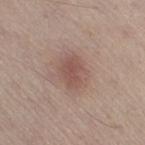| field | value |
|---|---|
| notes | no biopsy performed (imaged during a skin exam) |
| imaging modality | ~15 mm crop, total-body skin-cancer survey |
| tile lighting | white-light |
| automated metrics | an area of roughly 10 mm² and two-axis asymmetry of about 0.15; a mean CIELAB color near L≈52 a*≈18 b*≈23, about 8 CIELAB-L* units darker than the surrounding skin, and a normalized lesion–skin contrast near 6; border irregularity of about 1.5 on a 0–10 scale, internal color variation of about 2.5 on a 0–10 scale, and radial color variation of about 1 |
| body site | the right thigh |
| patient | male, in their 60s |